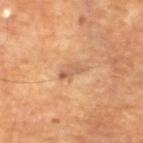The lesion was tiled from a total-body skin photograph and was not biopsied.
Located on the left lower leg.
The recorded lesion diameter is about 3 mm.
Cropped from a whole-body photographic skin survey; the tile spans about 15 mm.
The lesion-visualizer software estimated a lesion area of about 3.5 mm², an outline eccentricity of about 0.9 (0 = round, 1 = elongated), and a shape-asymmetry score of about 0.35 (0 = symmetric). The software also gave a mean CIELAB color near L≈58 a*≈21 b*≈34, a lesion–skin lightness drop of about 8, and a lesion-to-skin contrast of about 6 (normalized; higher = more distinct).
The tile uses cross-polarized illumination.
A male patient about 65 years old.